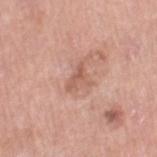<case>
  <biopsy_status>not biopsied; imaged during a skin examination</biopsy_status>
  <site>right thigh</site>
  <image>
    <source>total-body photography crop</source>
    <field_of_view_mm>15</field_of_view_mm>
  </image>
  <patient>
    <sex>female</sex>
    <age_approx>55</age_approx>
  </patient>
</case>